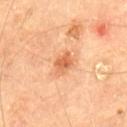Notes:
* workup · catalogued during a skin exam; not biopsied
* patient · male, roughly 65 years of age
* lesion diameter · ~3 mm (longest diameter)
* acquisition · total-body-photography crop, ~15 mm field of view
* anatomic site · the back